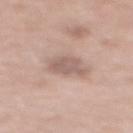Clinical summary: Imaged with white-light lighting. A 15 mm close-up extracted from a 3D total-body photography capture. About 3 mm across. The lesion is located on the upper back. Automated image analysis of the tile measured two-axis asymmetry of about 0.25. A female subject aged 68–72.